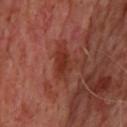Q: Is there a histopathology result?
A: total-body-photography surveillance lesion; no biopsy
Q: What is the imaging modality?
A: ~15 mm crop, total-body skin-cancer survey
Q: Lesion location?
A: the upper back
Q: Lesion size?
A: about 4 mm
Q: Patient demographics?
A: male, aged approximately 65
Q: What lighting was used for the tile?
A: cross-polarized